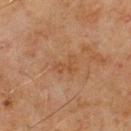notes: imaged on a skin check; not biopsied | image: 15 mm crop, total-body photography | body site: the upper back | subject: male, aged around 70.